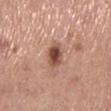<record>
  <biopsy_status>not biopsied; imaged during a skin examination</biopsy_status>
  <site>left lower leg</site>
  <lighting>white-light</lighting>
  <lesion_size>
    <long_diameter_mm_approx>3.0</long_diameter_mm_approx>
  </lesion_size>
  <image>
    <source>total-body photography crop</source>
    <field_of_view_mm>15</field_of_view_mm>
  </image>
  <patient>
    <sex>female</sex>
    <age_approx>65</age_approx>
  </patient>
</record>